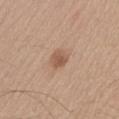{"biopsy_status": "not biopsied; imaged during a skin examination"}